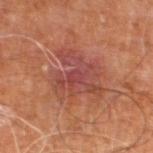Measured at roughly 6.5 mm in maximum diameter.
The lesion is on the right leg.
Imaged with cross-polarized lighting.
A roughly 15 mm field-of-view crop from a total-body skin photograph.
A male patient, aged 58–62.